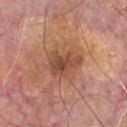Recorded during total-body skin imaging; not selected for excision or biopsy.
Cropped from a total-body skin-imaging series; the visible field is about 15 mm.
From the chest.
A male subject, in their mid-60s.